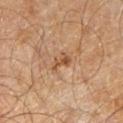Q: How was this image acquired?
A: ~15 mm crop, total-body skin-cancer survey
Q: Automated lesion metrics?
A: roughly 9 lightness units darker than nearby skin and a lesion-to-skin contrast of about 7 (normalized; higher = more distinct); an automated nevus-likeness rating near 0 out of 100 and lesion-presence confidence of about 100/100
Q: Who is the patient?
A: male, aged 58 to 62
Q: Where on the body is the lesion?
A: the leg